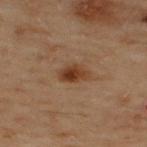Clinical impression: No biopsy was performed on this lesion — it was imaged during a full skin examination and was not determined to be concerning. Acquisition and patient details: A male subject aged approximately 50. From the upper back. Measured at roughly 3 mm in maximum diameter. Imaged with cross-polarized lighting. A 15 mm close-up extracted from a 3D total-body photography capture.